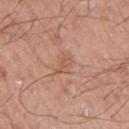Captured during whole-body skin photography for melanoma surveillance; the lesion was not biopsied.